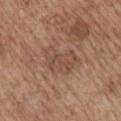Recorded during total-body skin imaging; not selected for excision or biopsy. This is a white-light tile. The total-body-photography lesion software estimated a lesion color around L≈49 a*≈20 b*≈26 in CIELAB and a lesion-to-skin contrast of about 5.5 (normalized; higher = more distinct). And it measured a border-irregularity rating of about 4/10 and radial color variation of about 1. The analysis additionally found lesion-presence confidence of about 100/100. The patient is a male aged 68 to 72. Cropped from a whole-body photographic skin survey; the tile spans about 15 mm. On the right upper arm.